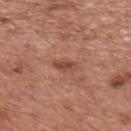Assessment:
The lesion was tiled from a total-body skin photograph and was not biopsied.
Clinical summary:
A male subject aged 68–72. This is a white-light tile. Cropped from a whole-body photographic skin survey; the tile spans about 15 mm. Automated tile analysis of the lesion measured an eccentricity of roughly 0.85. And it measured a lesion color around L≈48 a*≈25 b*≈28 in CIELAB and a lesion–skin lightness drop of about 9. The analysis additionally found a within-lesion color-variation index near 2/10 and peripheral color asymmetry of about 0.5. And it measured a nevus-likeness score of about 0/100 and a lesion-detection confidence of about 100/100. About 3 mm across. On the mid back.